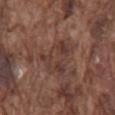No biopsy was performed on this lesion — it was imaged during a full skin examination and was not determined to be concerning. The patient is a male approximately 75 years of age. An algorithmic analysis of the crop reported a nevus-likeness score of about 0/100. On the back. This image is a 15 mm lesion crop taken from a total-body photograph.